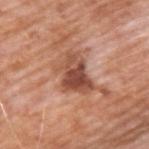Case summary:
– biopsy status: total-body-photography surveillance lesion; no biopsy
– illumination: white-light
– location: the upper back
– subject: male, approximately 60 years of age
– TBP lesion metrics: a mean CIELAB color near L≈50 a*≈25 b*≈31 and a normalized lesion–skin contrast near 9
– lesion diameter: ≈5 mm
– image: 15 mm crop, total-body photography